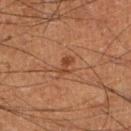Findings:
* notes: catalogued during a skin exam; not biopsied
* body site: the right lower leg
* tile lighting: cross-polarized
* automated metrics: a lesion color around L≈40 a*≈23 b*≈32 in CIELAB and a normalized border contrast of about 6.5
* patient: male, aged 58 to 62
* image: 15 mm crop, total-body photography